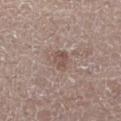Recorded during total-body skin imaging; not selected for excision or biopsy. A close-up tile cropped from a whole-body skin photograph, about 15 mm across. The patient is a male about 75 years old. From the left thigh.